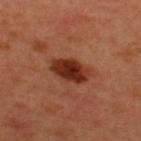Part of a total-body skin-imaging series; this lesion was reviewed on a skin check and was not flagged for biopsy.
A roughly 15 mm field-of-view crop from a total-body skin photograph.
Located on the upper back.
A male subject, roughly 50 years of age.